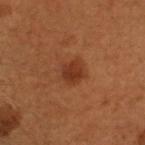Case summary:
• biopsy status · imaged on a skin check; not biopsied
• illumination · cross-polarized
• diameter · about 3 mm
• site · the head or neck
• subject · male, about 50 years old
• imaging modality · total-body-photography crop, ~15 mm field of view
• image-analysis metrics · a lesion area of about 5 mm², a shape eccentricity near 0.65, and a symmetry-axis asymmetry near 0.2; a within-lesion color-variation index near 2/10 and a peripheral color-asymmetry measure near 1; an automated nevus-likeness rating near 95 out of 100